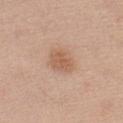Q: What is the imaging modality?
A: 15 mm crop, total-body photography
Q: Lesion location?
A: the chest
Q: Lesion size?
A: ~3.5 mm (longest diameter)
Q: What lighting was used for the tile?
A: white-light illumination
Q: Patient demographics?
A: male, about 30 years old